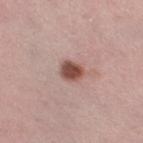No biopsy was performed on this lesion — it was imaged during a full skin examination and was not determined to be concerning. The tile uses white-light illumination. The recorded lesion diameter is about 3 mm. A close-up tile cropped from a whole-body skin photograph, about 15 mm across. On the leg. The total-body-photography lesion software estimated a mean CIELAB color near L≈50 a*≈22 b*≈25, a lesion–skin lightness drop of about 15, and a lesion-to-skin contrast of about 10.5 (normalized; higher = more distinct). The analysis additionally found a border-irregularity rating of about 1.5/10, a within-lesion color-variation index near 4.5/10, and peripheral color asymmetry of about 1.5. The analysis additionally found a detector confidence of about 100 out of 100 that the crop contains a lesion. A female patient in their mid-40s.